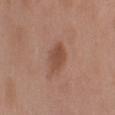Assessment:
The lesion was tiled from a total-body skin photograph and was not biopsied.
Acquisition and patient details:
A lesion tile, about 15 mm wide, cut from a 3D total-body photograph. The lesion is located on the arm. The tile uses white-light illumination. The total-body-photography lesion software estimated a lesion color around L≈48 a*≈21 b*≈29 in CIELAB and a lesion–skin lightness drop of about 9. And it measured a detector confidence of about 100 out of 100 that the crop contains a lesion. A male subject, aged around 50.